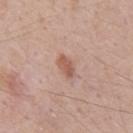{
  "biopsy_status": "not biopsied; imaged during a skin examination",
  "patient": {
    "sex": "male",
    "age_approx": 70
  },
  "lesion_size": {
    "long_diameter_mm_approx": 3.0
  },
  "image": {
    "source": "total-body photography crop",
    "field_of_view_mm": 15
  },
  "lighting": "white-light",
  "site": "chest"
}